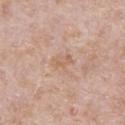A 15 mm crop from a total-body photograph taken for skin-cancer surveillance.
Captured under white-light illumination.
Automated image analysis of the tile measured a mean CIELAB color near L≈62 a*≈19 b*≈31. The analysis additionally found a border-irregularity index near 4/10 and a peripheral color-asymmetry measure near 1. It also reported a detector confidence of about 100 out of 100 that the crop contains a lesion.
The lesion is on the abdomen.
A male patient, about 60 years old.
Longest diameter approximately 3 mm.